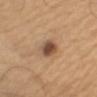Q: Who is the patient?
A: female, aged 58–62
Q: How was this image acquired?
A: 15 mm crop, total-body photography
Q: How was the tile lit?
A: white-light
Q: What did automated image analysis measure?
A: an area of roughly 6 mm², a shape eccentricity near 0.5, and a shape-asymmetry score of about 0.2 (0 = symmetric); border irregularity of about 2 on a 0–10 scale, a within-lesion color-variation index near 5/10, and radial color variation of about 1.5; a nevus-likeness score of about 85/100 and lesion-presence confidence of about 100/100
Q: How large is the lesion?
A: ~3 mm (longest diameter)
Q: Where on the body is the lesion?
A: the left upper arm